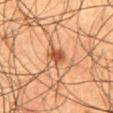Imaged during a routine full-body skin examination; the lesion was not biopsied and no histopathology is available. The tile uses cross-polarized illumination. Automated tile analysis of the lesion measured a border-irregularity rating of about 2.5/10, a within-lesion color-variation index near 3/10, and peripheral color asymmetry of about 1. A lesion tile, about 15 mm wide, cut from a 3D total-body photograph. A male subject, in their mid-50s. Located on the abdomen.